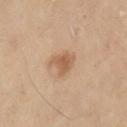Case summary:
• workup · catalogued during a skin exam; not biopsied
• patient · female, approximately 50 years of age
• body site · the right lower leg
• lighting · cross-polarized illumination
• lesion size · ≈3 mm
• acquisition · total-body-photography crop, ~15 mm field of view